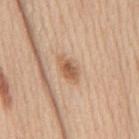{
  "image": {
    "source": "total-body photography crop",
    "field_of_view_mm": 15
  },
  "patient": {
    "sex": "male",
    "age_approx": 60
  },
  "site": "back"
}